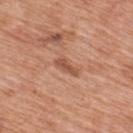Case summary:
– automated lesion analysis: a lesion area of about 3.5 mm², an eccentricity of roughly 0.95, and a symmetry-axis asymmetry near 0.35; a lesion color around L≈53 a*≈24 b*≈32 in CIELAB and a normalized border contrast of about 7; internal color variation of about 0.5 on a 0–10 scale; an automated nevus-likeness rating near 0 out of 100 and a lesion-detection confidence of about 100/100
– tile lighting: white-light
– patient: male, aged 68–72
– diameter: about 3 mm
– image source: ~15 mm tile from a whole-body skin photo
– body site: the upper back